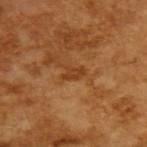{
  "biopsy_status": "not biopsied; imaged during a skin examination"
}